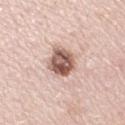<lesion>
  <biopsy_status>not biopsied; imaged during a skin examination</biopsy_status>
  <lesion_size>
    <long_diameter_mm_approx>4.5</long_diameter_mm_approx>
  </lesion_size>
  <image>
    <source>total-body photography crop</source>
    <field_of_view_mm>15</field_of_view_mm>
  </image>
  <patient>
    <sex>female</sex>
    <age_approx>50</age_approx>
  </patient>
  <site>arm</site>
</lesion>